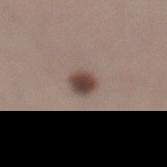Impression: Captured during whole-body skin photography for melanoma surveillance; the lesion was not biopsied. Context: Automated tile analysis of the lesion measured a lesion area of about 5.5 mm². It also reported a mean CIELAB color near L≈44 a*≈15 b*≈21 and about 14 CIELAB-L* units darker than the surrounding skin. And it measured an automated nevus-likeness rating near 100 out of 100 and lesion-presence confidence of about 100/100. Measured at roughly 3 mm in maximum diameter. Imaged with white-light lighting. A 15 mm crop from a total-body photograph taken for skin-cancer surveillance. The lesion is on the left thigh. A female patient aged around 40.